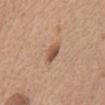Impression: Recorded during total-body skin imaging; not selected for excision or biopsy. Context: The patient is a female about 55 years old. The recorded lesion diameter is about 3 mm. Located on the abdomen. Automated image analysis of the tile measured an average lesion color of about L≈53 a*≈20 b*≈30 (CIELAB) and a normalized border contrast of about 8. The analysis additionally found a border-irregularity index near 2.5/10, a color-variation rating of about 3/10, and peripheral color asymmetry of about 1.5. And it measured an automated nevus-likeness rating near 80 out of 100 and a lesion-detection confidence of about 100/100. Captured under white-light illumination. A 15 mm crop from a total-body photograph taken for skin-cancer surveillance.